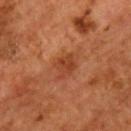Imaged during a routine full-body skin examination; the lesion was not biopsied and no histopathology is available. Imaged with cross-polarized lighting. The lesion is on the chest. A 15 mm close-up tile from a total-body photography series done for melanoma screening. A male patient, roughly 55 years of age.